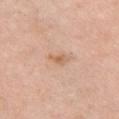Assessment:
Captured during whole-body skin photography for melanoma surveillance; the lesion was not biopsied.
Background:
A female patient in their mid-60s. A 15 mm close-up extracted from a 3D total-body photography capture. The tile uses white-light illumination. Automated tile analysis of the lesion measured a border-irregularity index near 3/10, internal color variation of about 2 on a 0–10 scale, and a peripheral color-asymmetry measure near 0.5. The software also gave a classifier nevus-likeness of about 0/100 and lesion-presence confidence of about 100/100. On the chest.